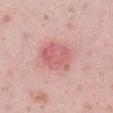biopsy status: imaged on a skin check; not biopsied
imaging modality: ~15 mm crop, total-body skin-cancer survey
lesion diameter: ~5 mm (longest diameter)
tile lighting: white-light illumination
anatomic site: the arm
subject: male, approximately 35 years of age